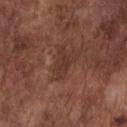Assessment: No biopsy was performed on this lesion — it was imaged during a full skin examination and was not determined to be concerning. Image and clinical context: Located on the chest. An algorithmic analysis of the crop reported an area of roughly 4.5 mm², an outline eccentricity of about 0.85 (0 = round, 1 = elongated), and a symmetry-axis asymmetry near 0.45. The analysis additionally found an average lesion color of about L≈34 a*≈20 b*≈24 (CIELAB), roughly 6 lightness units darker than nearby skin, and a normalized lesion–skin contrast near 6.5. The software also gave border irregularity of about 6 on a 0–10 scale, internal color variation of about 1 on a 0–10 scale, and a peripheral color-asymmetry measure near 0.5. The analysis additionally found an automated nevus-likeness rating near 0 out of 100 and lesion-presence confidence of about 95/100. A lesion tile, about 15 mm wide, cut from a 3D total-body photograph. A male subject, aged around 75. About 3.5 mm across.